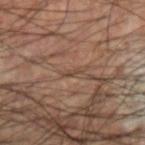Q: What did automated image analysis measure?
A: a lesion–skin lightness drop of about 7 and a lesion-to-skin contrast of about 6 (normalized; higher = more distinct); border irregularity of about 5 on a 0–10 scale
Q: What is the anatomic site?
A: the left forearm
Q: What is the lesion's diameter?
A: about 1.5 mm
Q: Illumination type?
A: cross-polarized
Q: Patient demographics?
A: male, aged approximately 45
Q: What is the imaging modality?
A: ~15 mm crop, total-body skin-cancer survey